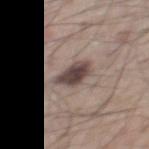– follow-up · catalogued during a skin exam; not biopsied
– tile lighting · white-light
– subject · male, aged around 75
– diameter · about 4 mm
– image · total-body-photography crop, ~15 mm field of view
– anatomic site · the chest